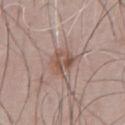The lesion was tiled from a total-body skin photograph and was not biopsied. The lesion's longest dimension is about 3 mm. The patient is a male roughly 65 years of age. The lesion is on the abdomen. A roughly 15 mm field-of-view crop from a total-body skin photograph. The tile uses white-light illumination.